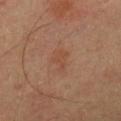<tbp_lesion>
<biopsy_status>not biopsied; imaged during a skin examination</biopsy_status>
<site>front of the torso</site>
<image>
  <source>total-body photography crop</source>
  <field_of_view_mm>15</field_of_view_mm>
</image>
<patient>
  <sex>male</sex>
  <age_approx>55</age_approx>
</patient>
</tbp_lesion>